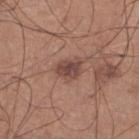workup — imaged on a skin check; not biopsied | patient — male, aged approximately 60 | body site — the left lower leg | acquisition — ~15 mm crop, total-body skin-cancer survey.